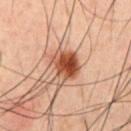workup: imaged on a skin check; not biopsied | lesion size: ~4 mm (longest diameter) | subject: male, about 60 years old | tile lighting: cross-polarized illumination | anatomic site: the chest | image source: ~15 mm crop, total-body skin-cancer survey | automated metrics: an outline eccentricity of about 0.55 (0 = round, 1 = elongated) and a symmetry-axis asymmetry near 0.25; a mean CIELAB color near L≈39 a*≈21 b*≈27, about 13 CIELAB-L* units darker than the surrounding skin, and a normalized lesion–skin contrast near 11.